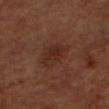Captured during whole-body skin photography for melanoma surveillance; the lesion was not biopsied. From the right forearm. The lesion-visualizer software estimated a mean CIELAB color near L≈26 a*≈19 b*≈24, a lesion–skin lightness drop of about 5, and a lesion-to-skin contrast of about 6 (normalized; higher = more distinct). The software also gave a border-irregularity rating of about 2.5/10, internal color variation of about 3.5 on a 0–10 scale, and radial color variation of about 1. A lesion tile, about 15 mm wide, cut from a 3D total-body photograph. This is a cross-polarized tile. The patient is a male aged approximately 65.